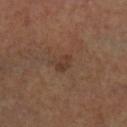Clinical impression:
Recorded during total-body skin imaging; not selected for excision or biopsy.
Image and clinical context:
The tile uses cross-polarized illumination. This image is a 15 mm lesion crop taken from a total-body photograph. Longest diameter approximately 2.5 mm. An algorithmic analysis of the crop reported a mean CIELAB color near L≈35 a*≈17 b*≈25, about 6 CIELAB-L* units darker than the surrounding skin, and a normalized lesion–skin contrast near 5.5. It also reported a nevus-likeness score of about 0/100. A male patient, about 60 years old. The lesion is on the left lower leg.